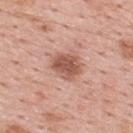Q: Lesion location?
A: the upper back
Q: How was this image acquired?
A: 15 mm crop, total-body photography
Q: What is the lesion's diameter?
A: about 3.5 mm
Q: What did automated image analysis measure?
A: a normalized border contrast of about 8.5; border irregularity of about 2 on a 0–10 scale, a within-lesion color-variation index near 3.5/10, and a peripheral color-asymmetry measure near 1; lesion-presence confidence of about 100/100
Q: How was the tile lit?
A: white-light
Q: Who is the patient?
A: male, about 55 years old